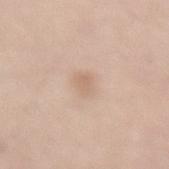Clinical impression: Captured during whole-body skin photography for melanoma surveillance; the lesion was not biopsied. Background: The patient is a male aged 73–77. An algorithmic analysis of the crop reported a classifier nevus-likeness of about 5/100 and lesion-presence confidence of about 100/100. A 15 mm close-up extracted from a 3D total-body photography capture. The recorded lesion diameter is about 3 mm. From the lower back.